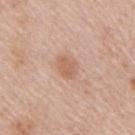Captured during whole-body skin photography for melanoma surveillance; the lesion was not biopsied. A lesion tile, about 15 mm wide, cut from a 3D total-body photograph. Captured under white-light illumination. On the arm. A female subject, about 40 years old.